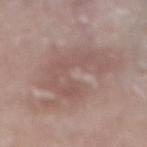follow-up=no biopsy performed (imaged during a skin exam)
lesion size=≈8.5 mm
subject=female, roughly 75 years of age
illumination=white-light
location=the right lower leg
acquisition=~15 mm crop, total-body skin-cancer survey
automated metrics=an area of roughly 30 mm², a shape eccentricity near 0.7, and a symmetry-axis asymmetry near 0.45; a mean CIELAB color near L≈54 a*≈17 b*≈22, a lesion–skin lightness drop of about 7, and a normalized border contrast of about 5; a border-irregularity rating of about 8.5/10, a color-variation rating of about 2.5/10, and radial color variation of about 1; a nevus-likeness score of about 0/100